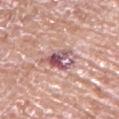Case summary:
- site — the arm
- patient — male, aged approximately 65
- acquisition — 15 mm crop, total-body photography